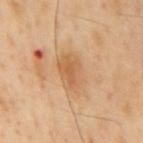Q: Was a biopsy performed?
A: imaged on a skin check; not biopsied
Q: What is the imaging modality?
A: total-body-photography crop, ~15 mm field of view
Q: Who is the patient?
A: male, aged around 55
Q: Where on the body is the lesion?
A: the mid back
Q: What lighting was used for the tile?
A: cross-polarized illumination
Q: Automated lesion metrics?
A: a mean CIELAB color near L≈61 a*≈20 b*≈38, a lesion–skin lightness drop of about 8, and a normalized border contrast of about 6; a border-irregularity rating of about 2/10 and radial color variation of about 1.5; a classifier nevus-likeness of about 0/100